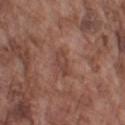Imaged during a routine full-body skin examination; the lesion was not biopsied and no histopathology is available. From the left upper arm. A roughly 15 mm field-of-view crop from a total-body skin photograph. Imaged with white-light lighting. The recorded lesion diameter is about 3 mm. A male patient roughly 75 years of age.